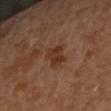<lesion>
<biopsy_status>not biopsied; imaged during a skin examination</biopsy_status>
<automated_metrics>
  <color_variation_0_10>3.0</color_variation_0_10>
  <peripheral_color_asymmetry>1.0</peripheral_color_asymmetry>
</automated_metrics>
<lighting>cross-polarized</lighting>
<image>
  <source>total-body photography crop</source>
  <field_of_view_mm>15</field_of_view_mm>
</image>
<site>right forearm</site>
<patient>
  <sex>female</sex>
  <age_approx>65</age_approx>
</patient>
</lesion>